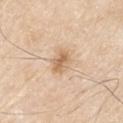The lesion was tiled from a total-body skin photograph and was not biopsied. The lesion-visualizer software estimated a footprint of about 5.5 mm² and an outline eccentricity of about 0.7 (0 = round, 1 = elongated). And it measured an average lesion color of about L≈66 a*≈17 b*≈35 (CIELAB), about 11 CIELAB-L* units darker than the surrounding skin, and a normalized lesion–skin contrast near 7. Cropped from a whole-body photographic skin survey; the tile spans about 15 mm. Approximately 3 mm at its widest. Imaged with white-light lighting. The lesion is on the right upper arm. A male patient, in their 80s.